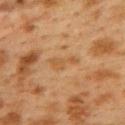follow-up: no biopsy performed (imaged during a skin exam)
image source: ~15 mm crop, total-body skin-cancer survey
lesion size: ~3.5 mm (longest diameter)
image-analysis metrics: a mean CIELAB color near L≈45 a*≈17 b*≈34, about 5 CIELAB-L* units darker than the surrounding skin, and a normalized border contrast of about 5
location: the upper back
subject: female, aged around 40